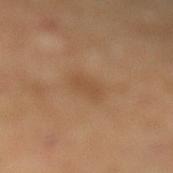image source: ~15 mm tile from a whole-body skin photo
location: the leg
patient: male, aged 63 to 67